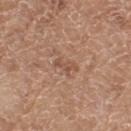* workup — catalogued during a skin exam; not biopsied
* patient — female, in their mid-70s
* lesion diameter — ~3 mm (longest diameter)
* tile lighting — white-light illumination
* body site — the left thigh
* automated metrics — a lesion area of about 3.5 mm² and a symmetry-axis asymmetry near 0.45; an average lesion color of about L≈52 a*≈20 b*≈29 (CIELAB), a lesion–skin lightness drop of about 7, and a normalized lesion–skin contrast near 5.5
* imaging modality — ~15 mm crop, total-body skin-cancer survey